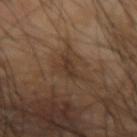Impression:
This lesion was catalogued during total-body skin photography and was not selected for biopsy.
Context:
A close-up tile cropped from a whole-body skin photograph, about 15 mm across. Automated image analysis of the tile measured a lesion area of about 5 mm². From the left forearm. About 3.5 mm across. This is a cross-polarized tile. The patient is a male aged approximately 65.